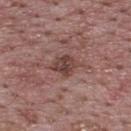biopsy status: imaged on a skin check; not biopsied
site: the upper back
imaging modality: total-body-photography crop, ~15 mm field of view
subject: male, in their 70s
lighting: white-light illumination
lesion diameter: ≈3 mm